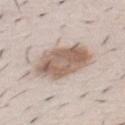Assessment: Imaged during a routine full-body skin examination; the lesion was not biopsied and no histopathology is available. Clinical summary: The lesion is located on the chest. A male subject, aged 48–52. A 15 mm close-up tile from a total-body photography series done for melanoma screening.